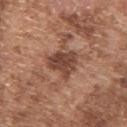Findings:
• follow-up — total-body-photography surveillance lesion; no biopsy
• location — the upper back
• image — ~15 mm crop, total-body skin-cancer survey
• subject — male, roughly 75 years of age
• lesion size — about 4 mm